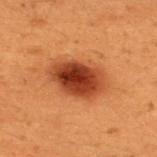Assessment: Recorded during total-body skin imaging; not selected for excision or biopsy. Acquisition and patient details: A male subject aged approximately 50. A region of skin cropped from a whole-body photographic capture, roughly 15 mm wide. The lesion is on the upper back.